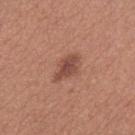{"biopsy_status": "not biopsied; imaged during a skin examination"}